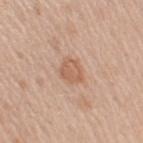{
  "biopsy_status": "not biopsied; imaged during a skin examination",
  "automated_metrics": {
    "area_mm2_approx": 6.0,
    "eccentricity": 0.65,
    "shape_asymmetry": 0.2,
    "nevus_likeness_0_100": 20,
    "lesion_detection_confidence_0_100": 100
  },
  "lighting": "white-light",
  "lesion_size": {
    "long_diameter_mm_approx": 3.0
  },
  "site": "arm",
  "image": {
    "source": "total-body photography crop",
    "field_of_view_mm": 15
  },
  "patient": {
    "sex": "female",
    "age_approx": 45
  }
}